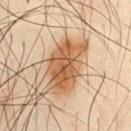Automated image analysis of the tile measured an outline eccentricity of about 0.85 (0 = round, 1 = elongated) and two-axis asymmetry of about 0.3. On the chest. A 15 mm close-up tile from a total-body photography series done for melanoma screening. The subject is a male aged 48–52. Captured under cross-polarized illumination.